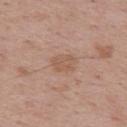Case summary:
• workup — imaged on a skin check; not biopsied
• image source — ~15 mm crop, total-body skin-cancer survey
• anatomic site — the back
• size — about 3.5 mm
• illumination — white-light illumination
• patient — male, aged 68 to 72